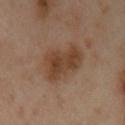No biopsy was performed on this lesion — it was imaged during a full skin examination and was not determined to be concerning. The lesion is on the arm. The tile uses cross-polarized illumination. Cropped from a total-body skin-imaging series; the visible field is about 15 mm. A female patient, aged around 40.